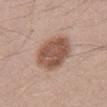Recorded during total-body skin imaging; not selected for excision or biopsy.
A male subject aged around 35.
From the mid back.
Captured under white-light illumination.
A region of skin cropped from a whole-body photographic capture, roughly 15 mm wide.